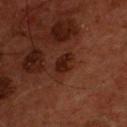Impression: Part of a total-body skin-imaging series; this lesion was reviewed on a skin check and was not flagged for biopsy. Image and clinical context: On the chest. An algorithmic analysis of the crop reported a lesion area of about 4.5 mm², an eccentricity of roughly 0.75, and two-axis asymmetry of about 0.2. It also reported an average lesion color of about L≈19 a*≈21 b*≈23 (CIELAB), roughly 8 lightness units darker than nearby skin, and a normalized lesion–skin contrast near 9. The software also gave a border-irregularity index near 2.5/10 and radial color variation of about 0.5. A close-up tile cropped from a whole-body skin photograph, about 15 mm across. Longest diameter approximately 3 mm. The patient is a male about 55 years old.